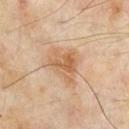The lesion was photographed on a routine skin check and not biopsied; there is no pathology result.
This is a cross-polarized tile.
The lesion is located on the chest.
A lesion tile, about 15 mm wide, cut from a 3D total-body photograph.
Measured at roughly 4 mm in maximum diameter.
A male patient aged around 65.
Automated tile analysis of the lesion measured an area of roughly 8 mm², a shape eccentricity near 0.65, and a symmetry-axis asymmetry near 0.4. The analysis additionally found about 9 CIELAB-L* units darker than the surrounding skin. The analysis additionally found a within-lesion color-variation index near 4/10 and radial color variation of about 1.5. It also reported a nevus-likeness score of about 0/100 and lesion-presence confidence of about 100/100.